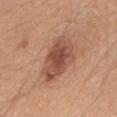workup = catalogued during a skin exam; not biopsied.